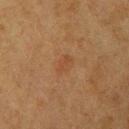<lesion>
  <biopsy_status>not biopsied; imaged during a skin examination</biopsy_status>
  <patient>
    <sex>female</sex>
    <age_approx>55</age_approx>
  </patient>
  <automated_metrics>
    <area_mm2_approx>3.0</area_mm2_approx>
    <shape_asymmetry>0.35</shape_asymmetry>
    <cielab_L>38</cielab_L>
    <cielab_a>18</cielab_a>
    <cielab_b>30</cielab_b>
    <vs_skin_darker_L>5.0</vs_skin_darker_L>
    <nevus_likeness_0_100>30</nevus_likeness_0_100>
    <lesion_detection_confidence_0_100>100</lesion_detection_confidence_0_100>
  </automated_metrics>
  <lighting>cross-polarized</lighting>
  <image>
    <source>total-body photography crop</source>
    <field_of_view_mm>15</field_of_view_mm>
  </image>
  <site>left upper arm</site>
</lesion>